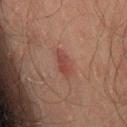Clinical impression: Part of a total-body skin-imaging series; this lesion was reviewed on a skin check and was not flagged for biopsy. Context: About 3.5 mm across. The lesion is located on the back. A roughly 15 mm field-of-view crop from a total-body skin photograph. The patient is a male aged around 45.